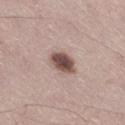This lesion was catalogued during total-body skin photography and was not selected for biopsy. A male patient, aged 43 to 47. About 3.5 mm across. The total-body-photography lesion software estimated a lesion-to-skin contrast of about 11.5 (normalized; higher = more distinct). The analysis additionally found border irregularity of about 1.5 on a 0–10 scale, a within-lesion color-variation index near 4.5/10, and radial color variation of about 1.5. It also reported an automated nevus-likeness rating near 95 out of 100 and a lesion-detection confidence of about 100/100. Captured under white-light illumination. From the right thigh. A 15 mm crop from a total-body photograph taken for skin-cancer surveillance.